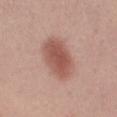This lesion was catalogued during total-body skin photography and was not selected for biopsy.
A female subject aged approximately 20.
This image is a 15 mm lesion crop taken from a total-body photograph.
The lesion is located on the left thigh.
Automated image analysis of the tile measured a lesion color around L≈54 a*≈23 b*≈26 in CIELAB, a lesion–skin lightness drop of about 12, and a normalized lesion–skin contrast near 8.